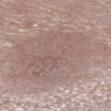Part of a total-body skin-imaging series; this lesion was reviewed on a skin check and was not flagged for biopsy.
The lesion's longest dimension is about 13 mm.
An algorithmic analysis of the crop reported an area of roughly 85 mm², an eccentricity of roughly 0.65, and a symmetry-axis asymmetry near 0.2.
A male subject, about 70 years old.
Located on the right lower leg.
A 15 mm close-up extracted from a 3D total-body photography capture.